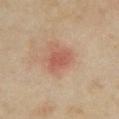Q: Is there a histopathology result?
A: no biopsy performed (imaged during a skin exam)
Q: Patient demographics?
A: female, aged 33–37
Q: Automated lesion metrics?
A: a mean CIELAB color near L≈53 a*≈24 b*≈29 and about 8 CIELAB-L* units darker than the surrounding skin; an automated nevus-likeness rating near 0 out of 100
Q: How was this image acquired?
A: ~15 mm tile from a whole-body skin photo
Q: What is the anatomic site?
A: the left upper arm